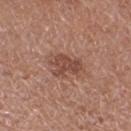Clinical impression:
Part of a total-body skin-imaging series; this lesion was reviewed on a skin check and was not flagged for biopsy.
Clinical summary:
A female subject in their 40s. An algorithmic analysis of the crop reported a mean CIELAB color near L≈47 a*≈22 b*≈26, a lesion–skin lightness drop of about 10, and a normalized lesion–skin contrast near 7.5. The software also gave a nevus-likeness score of about 55/100 and lesion-presence confidence of about 100/100. A 15 mm close-up extracted from a 3D total-body photography capture. On the right thigh. Longest diameter approximately 3.5 mm.